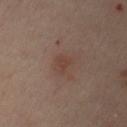Q: Was a biopsy performed?
A: no biopsy performed (imaged during a skin exam)
Q: What are the patient's age and sex?
A: female, aged approximately 45
Q: What is the imaging modality?
A: ~15 mm tile from a whole-body skin photo
Q: What did automated image analysis measure?
A: an area of roughly 3 mm² and a shape eccentricity near 0.8; internal color variation of about 0.5 on a 0–10 scale and peripheral color asymmetry of about 0
Q: What is the anatomic site?
A: the chest
Q: Lesion size?
A: about 2.5 mm